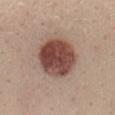tile lighting = white-light illumination; lesion diameter = ~5.5 mm (longest diameter); patient = female, aged 28 to 32; body site = the mid back; acquisition = 15 mm crop, total-body photography; histopathologic diagnosis = an intradermal melanocytic nevus — a benign skin lesion.